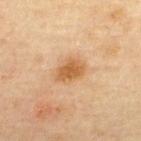workup — catalogued during a skin exam; not biopsied | patient — male, aged around 45 | acquisition — total-body-photography crop, ~15 mm field of view | illumination — cross-polarized | site — the upper back.